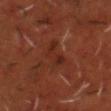Notes:
– biopsy status: no biopsy performed (imaged during a skin exam)
– image: ~15 mm crop, total-body skin-cancer survey
– anatomic site: the head or neck
– lesion diameter: about 4 mm
– lighting: cross-polarized
– patient: male, approximately 50 years of age